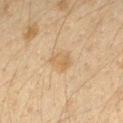Imaged during a routine full-body skin examination; the lesion was not biopsied and no histopathology is available.
The recorded lesion diameter is about 2.5 mm.
The patient is a female aged around 40.
This is a cross-polarized tile.
The total-body-photography lesion software estimated an average lesion color of about L≈52 a*≈13 b*≈32 (CIELAB), about 6 CIELAB-L* units darker than the surrounding skin, and a normalized lesion–skin contrast near 5.5. And it measured a classifier nevus-likeness of about 5/100.
A region of skin cropped from a whole-body photographic capture, roughly 15 mm wide.
From the right forearm.